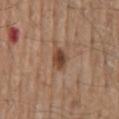{"biopsy_status": "not biopsied; imaged during a skin examination", "lesion_size": {"long_diameter_mm_approx": 3.0}, "site": "mid back", "patient": {"sex": "male", "age_approx": 70}, "automated_metrics": {"area_mm2_approx": 5.0, "eccentricity": 0.7, "cielab_L": 45, "cielab_a": 20, "cielab_b": 29, "border_irregularity_0_10": 1.5, "color_variation_0_10": 4.0, "peripheral_color_asymmetry": 1.0}, "lighting": "white-light", "image": {"source": "total-body photography crop", "field_of_view_mm": 15}}